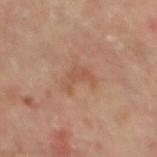Case summary:
- follow-up: imaged on a skin check; not biopsied
- diameter: ~4 mm (longest diameter)
- site: the back
- automated metrics: an area of roughly 6 mm², an outline eccentricity of about 0.85 (0 = round, 1 = elongated), and a shape-asymmetry score of about 0.55 (0 = symmetric); a nevus-likeness score of about 0/100 and a lesion-detection confidence of about 100/100
- tile lighting: cross-polarized illumination
- subject: male, aged 63 to 67
- image source: 15 mm crop, total-body photography